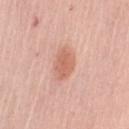Clinical impression:
Imaged during a routine full-body skin examination; the lesion was not biopsied and no histopathology is available.
Acquisition and patient details:
Captured under white-light illumination. A 15 mm close-up extracted from a 3D total-body photography capture. About 4 mm across. An algorithmic analysis of the crop reported an area of roughly 7.5 mm², an eccentricity of roughly 0.7, and a symmetry-axis asymmetry near 0.2. A female patient aged 63 to 67. Located on the right upper arm.